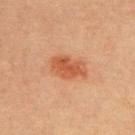The lesion was photographed on a routine skin check and not biopsied; there is no pathology result.
From the upper back.
A 15 mm close-up extracted from a 3D total-body photography capture.
The tile uses cross-polarized illumination.
A female subject aged 63 to 67.
The recorded lesion diameter is about 5 mm.